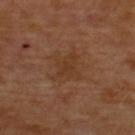biopsy_status: not biopsied; imaged during a skin examination
lesion_size:
  long_diameter_mm_approx: 4.0
patient:
  sex: male
  age_approx: 55
site: upper back
lighting: cross-polarized
image:
  source: total-body photography crop
  field_of_view_mm: 15
automated_metrics:
  nevus_likeness_0_100: 0